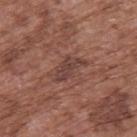This lesion was catalogued during total-body skin photography and was not selected for biopsy.
A male patient, aged 73–77.
A roughly 15 mm field-of-view crop from a total-body skin photograph.
The lesion is on the upper back.